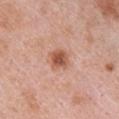A 15 mm close-up extracted from a 3D total-body photography capture. The patient is a female aged around 40. The lesion is on the chest. Approximately 2.5 mm at its widest. This is a white-light tile.